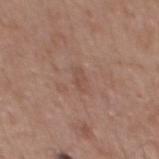Recorded during total-body skin imaging; not selected for excision or biopsy. A male subject, roughly 55 years of age. From the mid back. A 15 mm crop from a total-body photograph taken for skin-cancer surveillance. About 2.5 mm across.